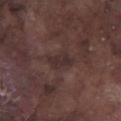Captured during whole-body skin photography for melanoma surveillance; the lesion was not biopsied. Approximately 3.5 mm at its widest. A male subject in their mid- to late 70s. Cropped from a whole-body photographic skin survey; the tile spans about 15 mm. On the leg.